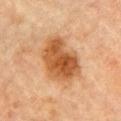Notes:
* biopsy status · no biopsy performed (imaged during a skin exam)
* subject · female, in their 70s
* TBP lesion metrics · a lesion-detection confidence of about 100/100
* image source · ~15 mm tile from a whole-body skin photo
* lesion size · about 6 mm
* tile lighting · cross-polarized
* anatomic site · the right upper arm